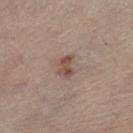| key | value |
|---|---|
| notes | catalogued during a skin exam; not biopsied |
| lesion size | ~2.5 mm (longest diameter) |
| lighting | white-light |
| image-analysis metrics | a lesion–skin lightness drop of about 10; a border-irregularity rating of about 3.5/10, internal color variation of about 2.5 on a 0–10 scale, and radial color variation of about 1 |
| body site | the right lower leg |
| subject | female, aged approximately 65 |
| image source | 15 mm crop, total-body photography |